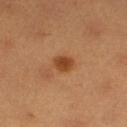size: ≈2.5 mm | subject: female, roughly 40 years of age | location: the left lower leg | imaging modality: 15 mm crop, total-body photography | illumination: cross-polarized illumination | automated lesion analysis: a footprint of about 4.5 mm², a shape eccentricity near 0.55, and two-axis asymmetry of about 0.2; a mean CIELAB color near L≈44 a*≈24 b*≈38, a lesion–skin lightness drop of about 11, and a lesion-to-skin contrast of about 8.5 (normalized; higher = more distinct); a border-irregularity index near 1.5/10, a within-lesion color-variation index near 2.5/10, and radial color variation of about 1; a detector confidence of about 100 out of 100 that the crop contains a lesion.